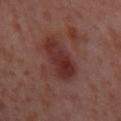notes: catalogued during a skin exam; not biopsied | patient: female, aged 53–57 | automated metrics: a lesion–skin lightness drop of about 10 and a lesion-to-skin contrast of about 9 (normalized; higher = more distinct); a color-variation rating of about 4.5/10 and a peripheral color-asymmetry measure near 1.5; a detector confidence of about 100 out of 100 that the crop contains a lesion | location: the right thigh | tile lighting: cross-polarized | lesion size: about 6.5 mm | image: total-body-photography crop, ~15 mm field of view.